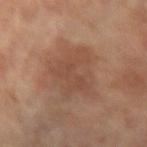Clinical impression:
The lesion was photographed on a routine skin check and not biopsied; there is no pathology result.
Acquisition and patient details:
Automated tile analysis of the lesion measured a lesion area of about 20 mm², an outline eccentricity of about 0.7 (0 = round, 1 = elongated), and a shape-asymmetry score of about 0.25 (0 = symmetric). The analysis additionally found a mean CIELAB color near L≈46 a*≈19 b*≈28 and a lesion-to-skin contrast of about 5.5 (normalized; higher = more distinct). The software also gave border irregularity of about 3.5 on a 0–10 scale and internal color variation of about 3 on a 0–10 scale. A close-up tile cropped from a whole-body skin photograph, about 15 mm across. From the arm. Captured under cross-polarized illumination. About 6 mm across. A female patient aged 63 to 67.